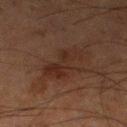  biopsy_status: not biopsied; imaged during a skin examination
  patient:
    sex: male
    age_approx: 65
  site: left lower leg
  lighting: cross-polarized
  automated_metrics:
    area_mm2_approx: 11.0
    eccentricity: 0.9
    shape_asymmetry: 0.4
    nevus_likeness_0_100: 5
  lesion_size:
    long_diameter_mm_approx: 5.5
  image:
    source: total-body photography crop
    field_of_view_mm: 15